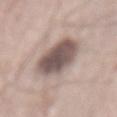Clinical impression: This lesion was catalogued during total-body skin photography and was not selected for biopsy. Clinical summary: A lesion tile, about 15 mm wide, cut from a 3D total-body photograph. On the mid back. The subject is a male aged around 65.